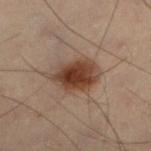Imaged during a routine full-body skin examination; the lesion was not biopsied and no histopathology is available.